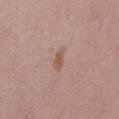notes: no biopsy performed (imaged during a skin exam) | tile lighting: white-light | diameter: ≈2.5 mm | subject: male, aged 53–57 | image: 15 mm crop, total-body photography | anatomic site: the lower back.